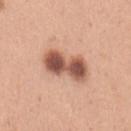The patient is a female roughly 30 years of age.
Approximately 5.5 mm at its widest.
The lesion-visualizer software estimated a footprint of about 14 mm², an eccentricity of roughly 0.85, and a shape-asymmetry score of about 0.15 (0 = symmetric). It also reported an average lesion color of about L≈55 a*≈23 b*≈29 (CIELAB), about 17 CIELAB-L* units darker than the surrounding skin, and a normalized border contrast of about 11. And it measured internal color variation of about 7 on a 0–10 scale. It also reported a nevus-likeness score of about 95/100 and lesion-presence confidence of about 100/100.
Imaged with white-light lighting.
The lesion is on the left upper arm.
Cropped from a total-body skin-imaging series; the visible field is about 15 mm.